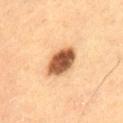Impression:
Captured during whole-body skin photography for melanoma surveillance; the lesion was not biopsied.
Background:
Imaged with cross-polarized lighting. A male patient approximately 60 years of age. The lesion is on the lower back. Measured at roughly 4.5 mm in maximum diameter. A region of skin cropped from a whole-body photographic capture, roughly 15 mm wide.